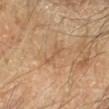Q: Is there a histopathology result?
A: no biopsy performed (imaged during a skin exam)
Q: What lighting was used for the tile?
A: cross-polarized
Q: Where on the body is the lesion?
A: the left forearm
Q: What are the patient's age and sex?
A: male, roughly 50 years of age
Q: Automated lesion metrics?
A: a border-irregularity rating of about 7/10, a within-lesion color-variation index near 0/10, and radial color variation of about 0
Q: How was this image acquired?
A: ~15 mm crop, total-body skin-cancer survey
Q: What is the lesion's diameter?
A: ~3 mm (longest diameter)